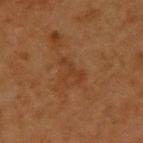Captured during whole-body skin photography for melanoma surveillance; the lesion was not biopsied. Measured at roughly 3.5 mm in maximum diameter. Imaged with cross-polarized lighting. Cropped from a whole-body photographic skin survey; the tile spans about 15 mm. Automated image analysis of the tile measured border irregularity of about 8.5 on a 0–10 scale, internal color variation of about 0 on a 0–10 scale, and radial color variation of about 0. The software also gave a classifier nevus-likeness of about 0/100. Located on the upper back. The subject is a female aged around 40.